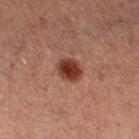biopsy status=imaged on a skin check; not biopsied | size=~3 mm (longest diameter) | site=the left lower leg | illumination=cross-polarized illumination | acquisition=~15 mm crop, total-body skin-cancer survey | subject=male, in their 60s.